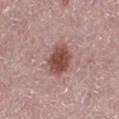Imaged during a routine full-body skin examination; the lesion was not biopsied and no histopathology is available. The lesion is on the leg. The patient is a female aged around 50. A close-up tile cropped from a whole-body skin photograph, about 15 mm across.